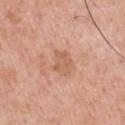Case summary:
- notes: total-body-photography surveillance lesion; no biopsy
- body site: the front of the torso
- lighting: white-light
- imaging modality: total-body-photography crop, ~15 mm field of view
- subject: male, aged 58–62